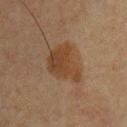Part of a total-body skin-imaging series; this lesion was reviewed on a skin check and was not flagged for biopsy.
The lesion is located on the chest.
Approximately 5 mm at its widest.
The total-body-photography lesion software estimated border irregularity of about 3.5 on a 0–10 scale, a color-variation rating of about 3/10, and radial color variation of about 1.
A 15 mm close-up tile from a total-body photography series done for melanoma screening.
The tile uses cross-polarized illumination.
A male patient, approximately 60 years of age.